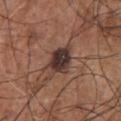Captured during whole-body skin photography for melanoma surveillance; the lesion was not biopsied. The total-body-photography lesion software estimated an average lesion color of about L≈34 a*≈17 b*≈20 (CIELAB) and a lesion-to-skin contrast of about 13.5 (normalized; higher = more distinct). And it measured a border-irregularity rating of about 2.5/10 and peripheral color asymmetry of about 1. The analysis additionally found a classifier nevus-likeness of about 10/100 and a detector confidence of about 100 out of 100 that the crop contains a lesion. Located on the chest. This is a white-light tile. A male patient, aged around 55. Approximately 3.5 mm at its widest. A 15 mm close-up extracted from a 3D total-body photography capture.